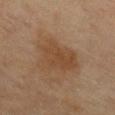Impression:
The lesion was tiled from a total-body skin photograph and was not biopsied.
Clinical summary:
From the chest. A roughly 15 mm field-of-view crop from a total-body skin photograph. Imaged with cross-polarized lighting. The patient is a female aged approximately 80.